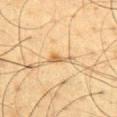<lesion>
<biopsy_status>not biopsied; imaged during a skin examination</biopsy_status>
<lighting>cross-polarized</lighting>
<patient>
  <sex>male</sex>
  <age_approx>60</age_approx>
</patient>
<site>right upper arm</site>
<lesion_size>
  <long_diameter_mm_approx>3.0</long_diameter_mm_approx>
</lesion_size>
<image>
  <source>total-body photography crop</source>
  <field_of_view_mm>15</field_of_view_mm>
</image>
</lesion>